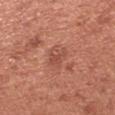notes — no biopsy performed (imaged during a skin exam)
patient — female, aged 48 to 52
body site — the left forearm
tile lighting — white-light illumination
image — ~15 mm crop, total-body skin-cancer survey
automated metrics — an area of roughly 5 mm² and two-axis asymmetry of about 0.35; an average lesion color of about L≈51 a*≈27 b*≈30 (CIELAB), roughly 7 lightness units darker than nearby skin, and a normalized lesion–skin contrast near 5.5; a border-irregularity rating of about 3.5/10 and radial color variation of about 1; lesion-presence confidence of about 100/100
lesion diameter — ~2.5 mm (longest diameter)